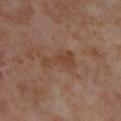Notes:
* biopsy status — no biopsy performed (imaged during a skin exam)
* patient — female, in their mid-50s
* diameter — ≈4 mm
* image — ~15 mm tile from a whole-body skin photo
* location — the right lower leg
* automated metrics — a mean CIELAB color near L≈43 a*≈19 b*≈29, a lesion–skin lightness drop of about 6, and a normalized lesion–skin contrast near 6; a classifier nevus-likeness of about 0/100 and a detector confidence of about 100 out of 100 that the crop contains a lesion
* illumination — cross-polarized